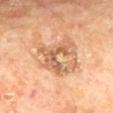<tbp_lesion>
<biopsy_status>not biopsied; imaged during a skin examination</biopsy_status>
<automated_metrics>
  <cielab_L>61</cielab_L>
  <cielab_a>22</cielab_a>
  <cielab_b>36</cielab_b>
  <vs_skin_darker_L>11.0</vs_skin_darker_L>
  <vs_skin_contrast_norm>7.0</vs_skin_contrast_norm>
  <peripheral_color_asymmetry>1.0</peripheral_color_asymmetry>
</automated_metrics>
<site>mid back</site>
<lesion_size>
  <long_diameter_mm_approx>5.5</long_diameter_mm_approx>
</lesion_size>
<image>
  <source>total-body photography crop</source>
  <field_of_view_mm>15</field_of_view_mm>
</image>
<lighting>cross-polarized</lighting>
<patient>
  <sex>male</sex>
  <age_approx>70</age_approx>
</patient>
</tbp_lesion>